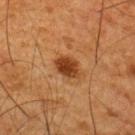The lesion was tiled from a total-body skin photograph and was not biopsied.
Longest diameter approximately 3.5 mm.
On the upper back.
Cropped from a total-body skin-imaging series; the visible field is about 15 mm.
The subject is a male aged 63 to 67.